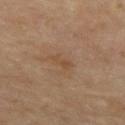The lesion was photographed on a routine skin check and not biopsied; there is no pathology result.
From the chest.
A female subject aged approximately 50.
About 2.5 mm across.
This is a cross-polarized tile.
A 15 mm close-up tile from a total-body photography series done for melanoma screening.
An algorithmic analysis of the crop reported a lesion area of about 3 mm², an outline eccentricity of about 0.9 (0 = round, 1 = elongated), and two-axis asymmetry of about 0.35. And it measured a border-irregularity index near 3.5/10 and a color-variation rating of about 0/10.